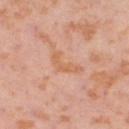Clinical impression:
This lesion was catalogued during total-body skin photography and was not selected for biopsy.
Image and clinical context:
Approximately 3.5 mm at its widest. The lesion is on the right thigh. A female patient aged 53–57. A lesion tile, about 15 mm wide, cut from a 3D total-body photograph. The tile uses cross-polarized illumination.